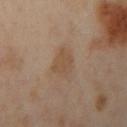Impression: Recorded during total-body skin imaging; not selected for excision or biopsy. Context: Automated image analysis of the tile measured a footprint of about 6 mm², an outline eccentricity of about 0.5 (0 = round, 1 = elongated), and a symmetry-axis asymmetry near 0.25. The analysis additionally found a border-irregularity rating of about 2.5/10, internal color variation of about 2 on a 0–10 scale, and a peripheral color-asymmetry measure near 1. The analysis additionally found a nevus-likeness score of about 10/100 and a detector confidence of about 100 out of 100 that the crop contains a lesion. From the arm. The patient is a female aged 38–42. A lesion tile, about 15 mm wide, cut from a 3D total-body photograph.